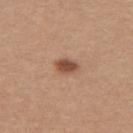notes — total-body-photography surveillance lesion; no biopsy | TBP lesion metrics — a mean CIELAB color near L≈49 a*≈22 b*≈30, a lesion–skin lightness drop of about 14, and a lesion-to-skin contrast of about 9.5 (normalized; higher = more distinct); a classifier nevus-likeness of about 95/100 | size — ≈2.5 mm | subject — female, in their 30s | anatomic site — the upper back | image — ~15 mm tile from a whole-body skin photo.